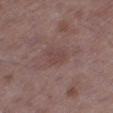<tbp_lesion>
<biopsy_status>not biopsied; imaged during a skin examination</biopsy_status>
<lighting>white-light</lighting>
<site>right thigh</site>
<patient>
  <sex>male</sex>
  <age_approx>50</age_approx>
</patient>
<image>
  <source>total-body photography crop</source>
  <field_of_view_mm>15</field_of_view_mm>
</image>
</tbp_lesion>